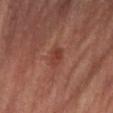notes: catalogued during a skin exam; not biopsied | subject: male, approximately 70 years of age | acquisition: ~15 mm tile from a whole-body skin photo | body site: the left lower leg.